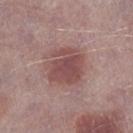Case summary:
* follow-up — catalogued during a skin exam; not biopsied
* site — the leg
* image — ~15 mm tile from a whole-body skin photo
* patient — male, aged approximately 75
* illumination — white-light
* diameter — ~4.5 mm (longest diameter)
* automated lesion analysis — a border-irregularity index near 3/10, internal color variation of about 3 on a 0–10 scale, and a peripheral color-asymmetry measure near 1; an automated nevus-likeness rating near 80 out of 100 and a detector confidence of about 100 out of 100 that the crop contains a lesion